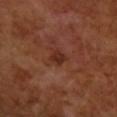The lesion was photographed on a routine skin check and not biopsied; there is no pathology result.
The lesion is located on the right forearm.
The subject is a female in their 50s.
A 15 mm close-up tile from a total-body photography series done for melanoma screening.
The tile uses cross-polarized illumination.
Measured at roughly 1.5 mm in maximum diameter.